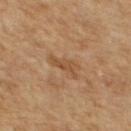Q: Is there a histopathology result?
A: no biopsy performed (imaged during a skin exam)
Q: Who is the patient?
A: female, roughly 60 years of age
Q: How was the tile lit?
A: cross-polarized
Q: What is the anatomic site?
A: the upper back
Q: Lesion size?
A: about 3 mm
Q: How was this image acquired?
A: ~15 mm crop, total-body skin-cancer survey